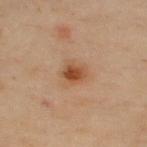Q: What is the lesion's diameter?
A: ≈2.5 mm
Q: What is the imaging modality?
A: ~15 mm tile from a whole-body skin photo
Q: Lesion location?
A: the chest
Q: What lighting was used for the tile?
A: cross-polarized illumination
Q: Patient demographics?
A: male, aged 48–52